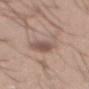Q: Was a biopsy performed?
A: total-body-photography surveillance lesion; no biopsy
Q: Illumination type?
A: white-light illumination
Q: How large is the lesion?
A: about 6.5 mm
Q: How was this image acquired?
A: ~15 mm tile from a whole-body skin photo
Q: What are the patient's age and sex?
A: male, roughly 40 years of age
Q: Lesion location?
A: the abdomen